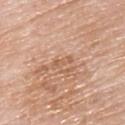The lesion was photographed on a routine skin check and not biopsied; there is no pathology result.